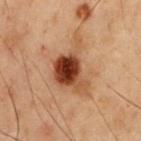Impression: Imaged during a routine full-body skin examination; the lesion was not biopsied and no histopathology is available. Context: The lesion is located on the front of the torso. A male patient, aged approximately 55. Cropped from a total-body skin-imaging series; the visible field is about 15 mm.